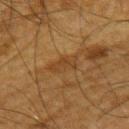A region of skin cropped from a whole-body photographic capture, roughly 15 mm wide. A male patient in their mid- to late 60s. Imaged with cross-polarized lighting. The lesion is on the upper back. About 4 mm across.